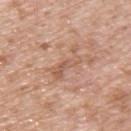Clinical impression:
Imaged during a routine full-body skin examination; the lesion was not biopsied and no histopathology is available.
Image and clinical context:
From the upper back. A male patient approximately 50 years of age. A 15 mm crop from a total-body photograph taken for skin-cancer surveillance. This is a white-light tile.